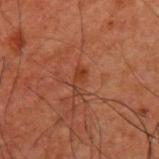Q: Was this lesion biopsied?
A: no biopsy performed (imaged during a skin exam)